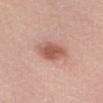Notes:
• follow-up — catalogued during a skin exam; not biopsied